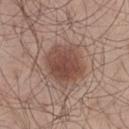Findings:
• workup — catalogued during a skin exam; not biopsied
• site — the left thigh
• lighting — white-light illumination
• lesion diameter — ~5 mm (longest diameter)
• automated metrics — a footprint of about 18 mm² and a shape eccentricity near 0.45; a lesion color around L≈47 a*≈20 b*≈25 in CIELAB and about 11 CIELAB-L* units darker than the surrounding skin; a peripheral color-asymmetry measure near 1; a nevus-likeness score of about 95/100 and a detector confidence of about 100 out of 100 that the crop contains a lesion
• patient — male, in their mid-50s
• acquisition — total-body-photography crop, ~15 mm field of view